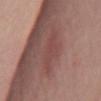notes: imaged on a skin check; not biopsied.